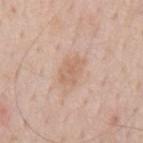No biopsy was performed on this lesion — it was imaged during a full skin examination and was not determined to be concerning.
Located on the mid back.
A male subject, in their 60s.
The lesion-visualizer software estimated a border-irregularity rating of about 2.5/10, a color-variation rating of about 2/10, and radial color variation of about 0.5. It also reported a nevus-likeness score of about 10/100 and a lesion-detection confidence of about 100/100.
This image is a 15 mm lesion crop taken from a total-body photograph.
Imaged with white-light lighting.